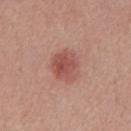Assessment:
The lesion was tiled from a total-body skin photograph and was not biopsied.
Clinical summary:
Captured under white-light illumination. The recorded lesion diameter is about 4 mm. Automated tile analysis of the lesion measured a border-irregularity index near 2/10, a color-variation rating of about 4/10, and peripheral color asymmetry of about 1. It also reported an automated nevus-likeness rating near 95 out of 100 and a lesion-detection confidence of about 100/100. Located on the mid back. A male patient, about 55 years old. Cropped from a whole-body photographic skin survey; the tile spans about 15 mm.